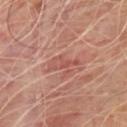automated metrics — an area of roughly 4 mm² and an eccentricity of roughly 0.95; an average lesion color of about L≈51 a*≈26 b*≈26 (CIELAB), about 7 CIELAB-L* units darker than the surrounding skin, and a normalized border contrast of about 5.5; border irregularity of about 6.5 on a 0–10 scale, internal color variation of about 1.5 on a 0–10 scale, and radial color variation of about 0.5; a classifier nevus-likeness of about 0/100
illumination — cross-polarized illumination
subject — male, aged approximately 70
site — the front of the torso
diameter — about 4 mm
image source — ~15 mm tile from a whole-body skin photo